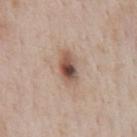Part of a total-body skin-imaging series; this lesion was reviewed on a skin check and was not flagged for biopsy. A male subject, aged around 65. About 4 mm across. On the front of the torso. This is a white-light tile. Automated image analysis of the tile measured an area of roughly 6.5 mm², an eccentricity of roughly 0.85, and two-axis asymmetry of about 0.2. The software also gave about 15 CIELAB-L* units darker than the surrounding skin and a lesion-to-skin contrast of about 10 (normalized; higher = more distinct). It also reported a nevus-likeness score of about 95/100 and lesion-presence confidence of about 100/100. This image is a 15 mm lesion crop taken from a total-body photograph.